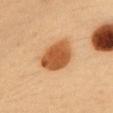Captured during whole-body skin photography for melanoma surveillance; the lesion was not biopsied. Imaged with cross-polarized lighting. This image is a 15 mm lesion crop taken from a total-body photograph. Located on the chest. A female subject, approximately 35 years of age. The lesion's longest dimension is about 5 mm.